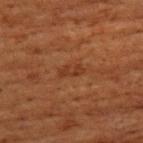Q: Lesion location?
A: the chest
Q: What is the imaging modality?
A: total-body-photography crop, ~15 mm field of view
Q: How was the tile lit?
A: cross-polarized illumination
Q: How large is the lesion?
A: about 2.5 mm
Q: What are the patient's age and sex?
A: female, about 80 years old